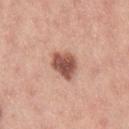This lesion was catalogued during total-body skin photography and was not selected for biopsy.
The lesion is located on the right thigh.
A lesion tile, about 15 mm wide, cut from a 3D total-body photograph.
The subject is a female aged 23 to 27.